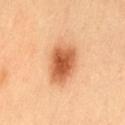Recorded during total-body skin imaging; not selected for excision or biopsy. Located on the lower back. The subject is a female roughly 50 years of age. Approximately 5 mm at its widest. This is a cross-polarized tile. Automated tile analysis of the lesion measured a lesion area of about 15 mm², a shape eccentricity near 0.75, and a shape-asymmetry score of about 0.2 (0 = symmetric). The analysis additionally found a border-irregularity index near 2/10, a color-variation rating of about 5.5/10, and peripheral color asymmetry of about 1.5. Cropped from a total-body skin-imaging series; the visible field is about 15 mm.